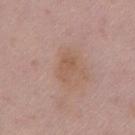image: ~15 mm tile from a whole-body skin photo; subject: female, approximately 50 years of age; anatomic site: the front of the torso; illumination: white-light illumination; lesion diameter: about 3.5 mm.